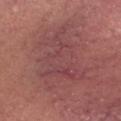| key | value |
|---|---|
| workup | imaged on a skin check; not biopsied |
| patient | male, approximately 65 years of age |
| imaging modality | ~15 mm crop, total-body skin-cancer survey |
| anatomic site | the head or neck |
| lesion size | about 9 mm |